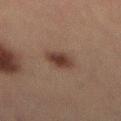Clinical impression: This lesion was catalogued during total-body skin photography and was not selected for biopsy. Background: Cropped from a whole-body photographic skin survey; the tile spans about 15 mm. The lesion is on the lower back. The total-body-photography lesion software estimated an average lesion color of about L≈28 a*≈14 b*≈19 (CIELAB), about 9 CIELAB-L* units darker than the surrounding skin, and a lesion-to-skin contrast of about 9 (normalized; higher = more distinct). The software also gave a color-variation rating of about 2.5/10 and peripheral color asymmetry of about 1. A male subject, roughly 50 years of age.